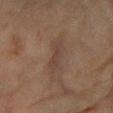Q: Is there a histopathology result?
A: no biopsy performed (imaged during a skin exam)
Q: How was this image acquired?
A: total-body-photography crop, ~15 mm field of view
Q: Lesion size?
A: ~3.5 mm (longest diameter)
Q: What are the patient's age and sex?
A: female, in their 80s
Q: How was the tile lit?
A: cross-polarized illumination
Q: Automated lesion metrics?
A: a lesion area of about 4.5 mm² and two-axis asymmetry of about 0.3; a mean CIELAB color near L≈34 a*≈14 b*≈21, a lesion–skin lightness drop of about 5, and a normalized lesion–skin contrast near 5; a within-lesion color-variation index near 1/10 and peripheral color asymmetry of about 0.5
Q: Where on the body is the lesion?
A: the right forearm